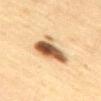| key | value |
|---|---|
| lesion size | ~5.5 mm (longest diameter) |
| image | 15 mm crop, total-body photography |
| patient | female, aged approximately 40 |
| body site | the back |